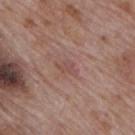Findings:
• follow-up: imaged on a skin check; not biopsied
• location: the mid back
• illumination: white-light
• subject: male, aged around 70
• imaging modality: total-body-photography crop, ~15 mm field of view
• image-analysis metrics: a lesion area of about 3.5 mm² and a shape eccentricity near 0.85; a lesion–skin lightness drop of about 6 and a lesion-to-skin contrast of about 4.5 (normalized; higher = more distinct); a nevus-likeness score of about 0/100 and lesion-presence confidence of about 100/100
• diameter: about 3 mm